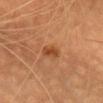Imaged during a routine full-body skin examination; the lesion was not biopsied and no histopathology is available.
Captured under cross-polarized illumination.
The patient is a female in their 50s.
The lesion-visualizer software estimated an outline eccentricity of about 0.75 (0 = round, 1 = elongated) and a shape-asymmetry score of about 0.35 (0 = symmetric).
The lesion's longest dimension is about 2.5 mm.
A 15 mm close-up tile from a total-body photography series done for melanoma screening.
Located on the head or neck.